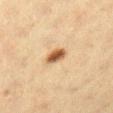Q: Was a biopsy performed?
A: catalogued during a skin exam; not biopsied
Q: What are the patient's age and sex?
A: female, aged 38 to 42
Q: How was the tile lit?
A: cross-polarized
Q: What is the imaging modality?
A: ~15 mm tile from a whole-body skin photo
Q: Lesion location?
A: the right lower leg